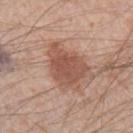image:
  source: total-body photography crop
  field_of_view_mm: 15
lesion_size:
  long_diameter_mm_approx: 5.5
site: left forearm
patient:
  sex: male
  age_approx: 35
lighting: white-light
diagnosis:
  histopathology: dysplastic (Clark) nevus
  malignancy: benign
  taxonomic_path:
    - Benign
    - Benign melanocytic proliferations
    - Nevus
    - Nevus, Atypical, Dysplastic, or Clark